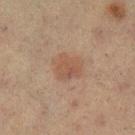Clinical impression: The lesion was tiled from a total-body skin photograph and was not biopsied. Image and clinical context: About 3.5 mm across. The lesion is on the left lower leg. This is a cross-polarized tile. The patient is a female aged around 55. A roughly 15 mm field-of-view crop from a total-body skin photograph.